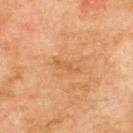The lesion was photographed on a routine skin check and not biopsied; there is no pathology result.
The lesion is located on the upper back.
A lesion tile, about 15 mm wide, cut from a 3D total-body photograph.
The tile uses cross-polarized illumination.
Automated image analysis of the tile measured an area of roughly 2.5 mm² and two-axis asymmetry of about 0.35. It also reported a mean CIELAB color near L≈48 a*≈21 b*≈36 and about 6 CIELAB-L* units darker than the surrounding skin. The software also gave a border-irregularity rating of about 6/10, internal color variation of about 0 on a 0–10 scale, and radial color variation of about 0.
Approximately 3.5 mm at its widest.
The subject is a male aged 73–77.